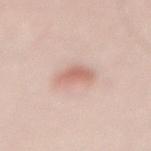biopsy_status: not biopsied; imaged during a skin examination
lighting: white-light
site: lower back
image:
  source: total-body photography crop
  field_of_view_mm: 15
lesion_size:
  long_diameter_mm_approx: 3.0
patient:
  sex: male
  age_approx: 70